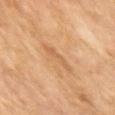The lesion is located on the arm.
A lesion tile, about 15 mm wide, cut from a 3D total-body photograph.
Automated tile analysis of the lesion measured a shape eccentricity near 0.95 and a shape-asymmetry score of about 0.4 (0 = symmetric). And it measured a nevus-likeness score of about 0/100 and lesion-presence confidence of about 100/100.
Longest diameter approximately 3.5 mm.
The patient is a female roughly 60 years of age.